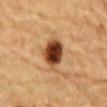No biopsy was performed on this lesion — it was imaged during a full skin examination and was not determined to be concerning.
A male subject, approximately 85 years of age or older.
A close-up tile cropped from a whole-body skin photograph, about 15 mm across.
From the abdomen.